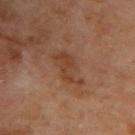Captured during whole-body skin photography for melanoma surveillance; the lesion was not biopsied.
A 15 mm crop from a total-body photograph taken for skin-cancer surveillance.
A male subject approximately 70 years of age.
Located on the upper back.